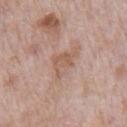Findings:
* notes — catalogued during a skin exam; not biopsied
* automated metrics — a mean CIELAB color near L≈57 a*≈19 b*≈27 and about 7 CIELAB-L* units darker than the surrounding skin
* subject — male, approximately 70 years of age
* tile lighting — white-light
* location — the chest
* image — ~15 mm crop, total-body skin-cancer survey